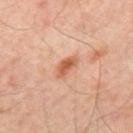Clinical impression: The lesion was tiled from a total-body skin photograph and was not biopsied. Acquisition and patient details: The tile uses cross-polarized illumination. A patient aged 53–57. Located on the mid back. A lesion tile, about 15 mm wide, cut from a 3D total-body photograph. About 3.5 mm across.